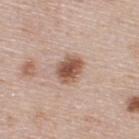The lesion was tiled from a total-body skin photograph and was not biopsied. Imaged with white-light lighting. A male patient, aged around 45. The lesion is located on the back. A 15 mm close-up tile from a total-body photography series done for melanoma screening.